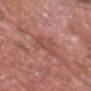Q: Is there a histopathology result?
A: catalogued during a skin exam; not biopsied
Q: Lesion location?
A: the head or neck
Q: Patient demographics?
A: male, aged 58 to 62
Q: What is the imaging modality?
A: total-body-photography crop, ~15 mm field of view
Q: What lighting was used for the tile?
A: white-light illumination
Q: Lesion size?
A: ≈5 mm
Q: What did automated image analysis measure?
A: a lesion area of about 5.5 mm² and an eccentricity of roughly 0.95; peripheral color asymmetry of about 0; an automated nevus-likeness rating near 0 out of 100 and a lesion-detection confidence of about 50/100